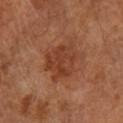Assessment:
Imaged during a routine full-body skin examination; the lesion was not biopsied and no histopathology is available.
Background:
A female subject, in their mid- to late 60s. The lesion is on the leg. A 15 mm close-up extracted from a 3D total-body photography capture.